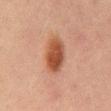Part of a total-body skin-imaging series; this lesion was reviewed on a skin check and was not flagged for biopsy. Captured under cross-polarized illumination. A 15 mm close-up tile from a total-body photography series done for melanoma screening. Approximately 4.5 mm at its widest. From the mid back. The subject is a male approximately 65 years of age.